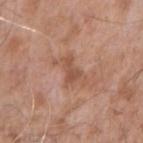The recorded lesion diameter is about 4.5 mm.
A close-up tile cropped from a whole-body skin photograph, about 15 mm across.
The subject is a male aged 73–77.
Located on the right forearm.